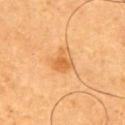Clinical impression:
The lesion was tiled from a total-body skin photograph and was not biopsied.
Context:
A 15 mm close-up extracted from a 3D total-body photography capture. On the right upper arm. A male patient aged around 65.